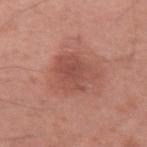Clinical impression: Imaged during a routine full-body skin examination; the lesion was not biopsied and no histopathology is available. Image and clinical context: A close-up tile cropped from a whole-body skin photograph, about 15 mm across. The lesion's longest dimension is about 4.5 mm. The tile uses white-light illumination. The lesion is located on the left upper arm. The total-body-photography lesion software estimated a lesion area of about 14 mm², an eccentricity of roughly 0.55, and two-axis asymmetry of about 0.25. It also reported peripheral color asymmetry of about 1. The subject is a male in their mid- to late 30s.